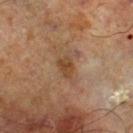Notes:
– notes — no biopsy performed (imaged during a skin exam)
– diameter — about 3 mm
– lighting — cross-polarized
– imaging modality — ~15 mm crop, total-body skin-cancer survey
– automated metrics — an area of roughly 4 mm², an eccentricity of roughly 0.8, and a symmetry-axis asymmetry near 0.25; a normalized lesion–skin contrast near 7.5; a border-irregularity rating of about 2.5/10 and a peripheral color-asymmetry measure near 0.5; an automated nevus-likeness rating near 5 out of 100 and a lesion-detection confidence of about 100/100
– subject — male, aged around 70
– site — the right lower leg